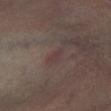Case summary:
– follow-up — no biopsy performed (imaged during a skin exam)
– illumination — cross-polarized
– subject — male, aged around 65
– imaging modality — ~15 mm crop, total-body skin-cancer survey
– body site — the right lower leg
– automated lesion analysis — a lesion area of about 2.5 mm², an eccentricity of roughly 0.9, and two-axis asymmetry of about 0.3; a border-irregularity index near 3.5/10 and peripheral color asymmetry of about 0; a detector confidence of about 95 out of 100 that the crop contains a lesion
– lesion diameter — ~2.5 mm (longest diameter)